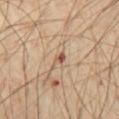follow-up — imaged on a skin check; not biopsied
location — the abdomen
image source — 15 mm crop, total-body photography
diameter — ≈2.5 mm
TBP lesion metrics — a peripheral color-asymmetry measure near 1; a classifier nevus-likeness of about 15/100 and lesion-presence confidence of about 95/100
illumination — cross-polarized illumination
subject — male, in their 70s